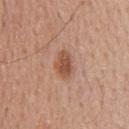biopsy status = total-body-photography surveillance lesion; no biopsy
location = the back
patient = male, approximately 60 years of age
imaging modality = total-body-photography crop, ~15 mm field of view